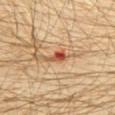biopsy status — total-body-photography surveillance lesion; no biopsy
anatomic site — the abdomen
size — ≈3.5 mm
tile lighting — cross-polarized illumination
subject — male, aged around 30
TBP lesion metrics — a lesion area of about 4 mm², a shape eccentricity near 0.9, and a symmetry-axis asymmetry near 0.5; a normalized border contrast of about 9; a color-variation rating of about 8.5/10 and peripheral color asymmetry of about 4; a detector confidence of about 100 out of 100 that the crop contains a lesion
image source — total-body-photography crop, ~15 mm field of view